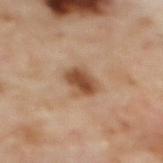The lesion was tiled from a total-body skin photograph and was not biopsied. The lesion's longest dimension is about 3 mm. The patient is a female approximately 60 years of age. Located on the back. A lesion tile, about 15 mm wide, cut from a 3D total-body photograph. Imaged with cross-polarized lighting. The lesion-visualizer software estimated a lesion color around L≈49 a*≈21 b*≈33 in CIELAB and a lesion–skin lightness drop of about 13. The analysis additionally found a border-irregularity index near 2/10, internal color variation of about 4 on a 0–10 scale, and a peripheral color-asymmetry measure near 1.